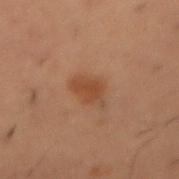The lesion-visualizer software estimated a lesion color around L≈35 a*≈18 b*≈27 in CIELAB, a lesion–skin lightness drop of about 7, and a normalized border contrast of about 6.5. And it measured a border-irregularity rating of about 3.5/10, a color-variation rating of about 1/10, and peripheral color asymmetry of about 0.5. The software also gave a nevus-likeness score of about 90/100. This image is a 15 mm lesion crop taken from a total-body photograph. This is a cross-polarized tile. From the front of the torso. A male subject aged around 50.